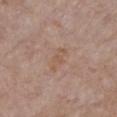Q: Was a biopsy performed?
A: imaged on a skin check; not biopsied
Q: Patient demographics?
A: female, in their mid- to late 80s or older
Q: Lesion location?
A: the chest
Q: What is the lesion's diameter?
A: about 3.5 mm
Q: How was the tile lit?
A: white-light illumination
Q: What kind of image is this?
A: total-body-photography crop, ~15 mm field of view